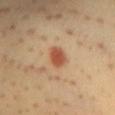No biopsy was performed on this lesion — it was imaged during a full skin examination and was not determined to be concerning. A female subject aged 28–32. Cropped from a total-body skin-imaging series; the visible field is about 15 mm. Imaged with cross-polarized lighting. On the front of the torso. The lesion-visualizer software estimated a mean CIELAB color near L≈53 a*≈26 b*≈36, roughly 13 lightness units darker than nearby skin, and a lesion-to-skin contrast of about 9 (normalized; higher = more distinct). The analysis additionally found an automated nevus-likeness rating near 100 out of 100 and a detector confidence of about 100 out of 100 that the crop contains a lesion. About 2.5 mm across.